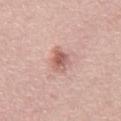notes: no biopsy performed (imaged during a skin exam) | lesion diameter: about 3.5 mm | subject: male, in their mid- to late 40s | image: total-body-photography crop, ~15 mm field of view | location: the abdomen | tile lighting: white-light illumination.